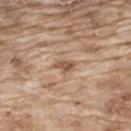Captured during whole-body skin photography for melanoma surveillance; the lesion was not biopsied.
This is a white-light tile.
A male subject aged around 65.
Longest diameter approximately 2.5 mm.
The total-body-photography lesion software estimated a symmetry-axis asymmetry near 0.35. And it measured a mean CIELAB color near L≈54 a*≈18 b*≈30 and roughly 11 lightness units darker than nearby skin.
On the upper back.
A lesion tile, about 15 mm wide, cut from a 3D total-body photograph.